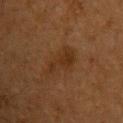Impression: Captured during whole-body skin photography for melanoma surveillance; the lesion was not biopsied. Acquisition and patient details: Cropped from a total-body skin-imaging series; the visible field is about 15 mm. The patient is a male roughly 65 years of age. Automated tile analysis of the lesion measured an average lesion color of about L≈25 a*≈16 b*≈27 (CIELAB) and about 5 CIELAB-L* units darker than the surrounding skin. The software also gave a classifier nevus-likeness of about 30/100. From the upper back. This is a cross-polarized tile.